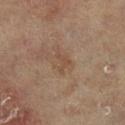Imaged during a routine full-body skin examination; the lesion was not biopsied and no histopathology is available. On the left lower leg. Cropped from a total-body skin-imaging series; the visible field is about 15 mm. A female subject, aged around 80.